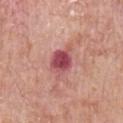workup: no biopsy performed (imaged during a skin exam)
lesion size: ~4.5 mm (longest diameter)
illumination: white-light
patient: male, roughly 65 years of age
body site: the chest
acquisition: ~15 mm tile from a whole-body skin photo
image-analysis metrics: an outline eccentricity of about 0.8 (0 = round, 1 = elongated); border irregularity of about 3.5 on a 0–10 scale, a color-variation rating of about 5.5/10, and a peripheral color-asymmetry measure near 2; a detector confidence of about 100 out of 100 that the crop contains a lesion